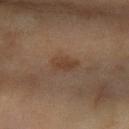Case summary:
- notes: catalogued during a skin exam; not biopsied
- subject: female, aged 58 to 62
- automated metrics: a lesion area of about 3.5 mm², an eccentricity of roughly 0.85, and two-axis asymmetry of about 0.25; a border-irregularity index near 2.5/10, a within-lesion color-variation index near 1.5/10, and a peripheral color-asymmetry measure near 0.5; a detector confidence of about 100 out of 100 that the crop contains a lesion
- body site: the arm
- lesion diameter: ~3 mm (longest diameter)
- acquisition: total-body-photography crop, ~15 mm field of view